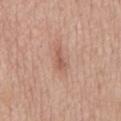A male patient aged approximately 65. A 15 mm close-up tile from a total-body photography series done for melanoma screening. Located on the mid back. The total-body-photography lesion software estimated an area of roughly 3 mm² and a symmetry-axis asymmetry near 0.3. The analysis additionally found a lesion color around L≈57 a*≈22 b*≈28 in CIELAB, about 9 CIELAB-L* units darker than the surrounding skin, and a lesion-to-skin contrast of about 6 (normalized; higher = more distinct). It also reported a border-irregularity rating of about 3.5/10, a within-lesion color-variation index near 1/10, and a peripheral color-asymmetry measure near 0.5. Approximately 3 mm at its widest. This is a white-light tile.